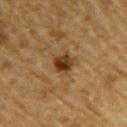Imaged during a routine full-body skin examination; the lesion was not biopsied and no histopathology is available. The lesion is located on the right upper arm. A 15 mm crop from a total-body photograph taken for skin-cancer surveillance. About 2.5 mm across. A male subject, aged 83 or over.